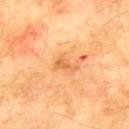A roughly 15 mm field-of-view crop from a total-body skin photograph.
This is a cross-polarized tile.
A male patient, aged 73–77.
The lesion is located on the upper back.
The total-body-photography lesion software estimated a border-irregularity index near 5.5/10 and radial color variation of about 0. And it measured lesion-presence confidence of about 100/100.
The recorded lesion diameter is about 3 mm.